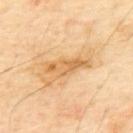biopsy_status: not biopsied; imaged during a skin examination
site: mid back
automated_metrics:
  area_mm2_approx: 8.5
  eccentricity: 0.95
  shape_asymmetry: 0.3
  nevus_likeness_0_100: 0
  lesion_detection_confidence_0_100: 100
image:
  source: total-body photography crop
  field_of_view_mm: 15
lesion_size:
  long_diameter_mm_approx: 5.5
patient:
  sex: male
  age_approx: 65